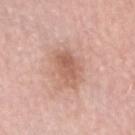Q: Was this lesion biopsied?
A: imaged on a skin check; not biopsied
Q: What did automated image analysis measure?
A: a lesion area of about 9.5 mm², an eccentricity of roughly 0.8, and a symmetry-axis asymmetry near 0.3; a border-irregularity rating of about 3/10, internal color variation of about 4 on a 0–10 scale, and a peripheral color-asymmetry measure near 1.5; lesion-presence confidence of about 100/100
Q: Patient demographics?
A: male, in their mid-60s
Q: Where on the body is the lesion?
A: the head or neck
Q: How large is the lesion?
A: ≈4.5 mm
Q: How was this image acquired?
A: ~15 mm tile from a whole-body skin photo
Q: What lighting was used for the tile?
A: white-light illumination